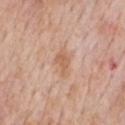Q: Patient demographics?
A: male, aged 58 to 62
Q: What is the anatomic site?
A: the chest
Q: How was this image acquired?
A: total-body-photography crop, ~15 mm field of view
Q: Illumination type?
A: white-light illumination
Q: What did automated image analysis measure?
A: a footprint of about 7 mm² and an eccentricity of roughly 0.75; a mean CIELAB color near L≈62 a*≈20 b*≈32, about 7 CIELAB-L* units darker than the surrounding skin, and a normalized border contrast of about 6; a border-irregularity index near 3/10, a within-lesion color-variation index near 2.5/10, and a peripheral color-asymmetry measure near 1; a classifier nevus-likeness of about 0/100 and a lesion-detection confidence of about 100/100